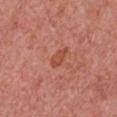Measured at roughly 3 mm in maximum diameter. This image is a 15 mm lesion crop taken from a total-body photograph. This is a white-light tile. The lesion-visualizer software estimated a footprint of about 3 mm², a shape eccentricity near 0.85, and a shape-asymmetry score of about 0.3 (0 = symmetric). The software also gave a lesion color around L≈49 a*≈30 b*≈33 in CIELAB, a lesion–skin lightness drop of about 8, and a lesion-to-skin contrast of about 6.5 (normalized; higher = more distinct). And it measured border irregularity of about 3 on a 0–10 scale, a color-variation rating of about 1.5/10, and peripheral color asymmetry of about 0.5. The software also gave a nevus-likeness score of about 5/100 and lesion-presence confidence of about 100/100. On the chest. The subject is a female aged approximately 60.